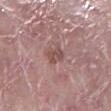Clinical impression: The lesion was photographed on a routine skin check and not biopsied; there is no pathology result. Image and clinical context: A roughly 15 mm field-of-view crop from a total-body skin photograph. The lesion is on the right lower leg. The patient is a female approximately 60 years of age. The recorded lesion diameter is about 2.5 mm.